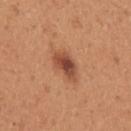Q: Was this lesion biopsied?
A: catalogued during a skin exam; not biopsied
Q: What kind of image is this?
A: total-body-photography crop, ~15 mm field of view
Q: What lighting was used for the tile?
A: white-light illumination
Q: What are the patient's age and sex?
A: female, aged approximately 45
Q: What is the lesion's diameter?
A: ≈4 mm
Q: What is the anatomic site?
A: the left upper arm